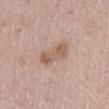Findings:
- workup: total-body-photography surveillance lesion; no biopsy
- subject: female, aged 63 to 67
- location: the abdomen
- image source: ~15 mm crop, total-body skin-cancer survey